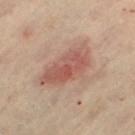A 15 mm crop from a total-body photograph taken for skin-cancer surveillance.
On the leg.
Automated tile analysis of the lesion measured a lesion area of about 16 mm² and two-axis asymmetry of about 0.25. And it measured a lesion color around L≈54 a*≈23 b*≈26 in CIELAB, roughly 10 lightness units darker than nearby skin, and a lesion-to-skin contrast of about 7 (normalized; higher = more distinct). And it measured a border-irregularity index near 3/10, a color-variation rating of about 5/10, and radial color variation of about 1.5. The analysis additionally found a nevus-likeness score of about 30/100 and a detector confidence of about 100 out of 100 that the crop contains a lesion.
The subject is a female aged approximately 60.
Captured under cross-polarized illumination.
The recorded lesion diameter is about 6.5 mm.